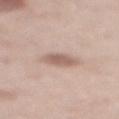workup: catalogued during a skin exam; not biopsied
location: the upper back
automated lesion analysis: an area of roughly 5.5 mm², an outline eccentricity of about 0.7 (0 = round, 1 = elongated), and a symmetry-axis asymmetry near 0.2; an average lesion color of about L≈60 a*≈17 b*≈24 (CIELAB), about 11 CIELAB-L* units darker than the surrounding skin, and a lesion-to-skin contrast of about 7 (normalized; higher = more distinct)
patient: female, in their mid-60s
acquisition: ~15 mm crop, total-body skin-cancer survey
lighting: white-light illumination